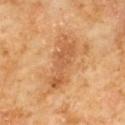Q: Is there a histopathology result?
A: no biopsy performed (imaged during a skin exam)
Q: Where on the body is the lesion?
A: the chest
Q: What is the lesion's diameter?
A: about 7 mm
Q: What lighting was used for the tile?
A: cross-polarized
Q: What are the patient's age and sex?
A: male, about 60 years old
Q: Automated lesion metrics?
A: an area of roughly 17 mm²; a border-irregularity rating of about 4.5/10 and peripheral color asymmetry of about 1.5
Q: How was this image acquired?
A: ~15 mm tile from a whole-body skin photo